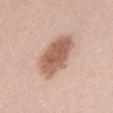biopsy status — catalogued during a skin exam; not biopsied | lighting — white-light | acquisition — total-body-photography crop, ~15 mm field of view | diameter — ≈6.5 mm | subject — male, roughly 25 years of age | site — the abdomen | image-analysis metrics — an automated nevus-likeness rating near 100 out of 100.